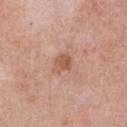Assessment: Part of a total-body skin-imaging series; this lesion was reviewed on a skin check and was not flagged for biopsy. Context: From the chest. Captured under white-light illumination. A male subject, aged 53 to 57. The lesion-visualizer software estimated roughly 9 lightness units darker than nearby skin. The analysis additionally found a border-irregularity index near 2.5/10 and a peripheral color-asymmetry measure near 1. The recorded lesion diameter is about 2.5 mm. A 15 mm crop from a total-body photograph taken for skin-cancer surveillance.